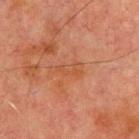Case summary:
- notes: no biopsy performed (imaged during a skin exam)
- imaging modality: ~15 mm tile from a whole-body skin photo
- tile lighting: cross-polarized illumination
- location: the chest
- image-analysis metrics: a classifier nevus-likeness of about 0/100 and lesion-presence confidence of about 100/100
- subject: male, aged approximately 70
- diameter: about 3.5 mm